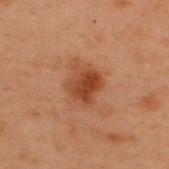Recorded during total-body skin imaging; not selected for excision or biopsy.
A region of skin cropped from a whole-body photographic capture, roughly 15 mm wide.
This is a cross-polarized tile.
Automated image analysis of the tile measured an average lesion color of about L≈36 a*≈23 b*≈29 (CIELAB), roughly 9 lightness units darker than nearby skin, and a normalized border contrast of about 8.5. The analysis additionally found a color-variation rating of about 4.5/10 and radial color variation of about 1.5. It also reported a classifier nevus-likeness of about 95/100 and lesion-presence confidence of about 100/100.
Measured at roughly 3.5 mm in maximum diameter.
The lesion is on the upper back.
The subject is a male roughly 55 years of age.